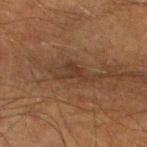Part of a total-body skin-imaging series; this lesion was reviewed on a skin check and was not flagged for biopsy. The lesion is on the left lower leg. The tile uses cross-polarized illumination. A 15 mm crop from a total-body photograph taken for skin-cancer surveillance. A male subject aged around 50. The recorded lesion diameter is about 5.5 mm.